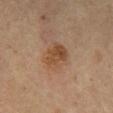No biopsy was performed on this lesion — it was imaged during a full skin examination and was not determined to be concerning.
The lesion is on the front of the torso.
The patient is a male roughly 65 years of age.
This image is a 15 mm lesion crop taken from a total-body photograph.